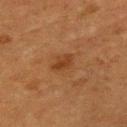A 15 mm close-up tile from a total-body photography series done for melanoma screening.
The patient is a male aged approximately 75.
On the chest.